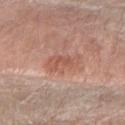follow-up: imaged on a skin check; not biopsied | site: the right forearm | imaging modality: ~15 mm crop, total-body skin-cancer survey | diameter: ≈4 mm | lighting: white-light | subject: female, about 60 years old.